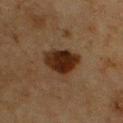Imaged during a routine full-body skin examination; the lesion was not biopsied and no histopathology is available.
The subject is a male in their mid-70s.
From the chest.
Approximately 4.5 mm at its widest.
The total-body-photography lesion software estimated a lesion–skin lightness drop of about 12. The software also gave a lesion-detection confidence of about 100/100.
This is a cross-polarized tile.
This image is a 15 mm lesion crop taken from a total-body photograph.